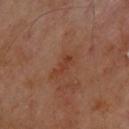Q: What did automated image analysis measure?
A: a footprint of about 3 mm², an eccentricity of roughly 0.9, and a symmetry-axis asymmetry near 0.45; an average lesion color of about L≈38 a*≈24 b*≈30 (CIELAB), about 6 CIELAB-L* units darker than the surrounding skin, and a normalized border contrast of about 6; border irregularity of about 5 on a 0–10 scale, internal color variation of about 0 on a 0–10 scale, and peripheral color asymmetry of about 0
Q: How was this image acquired?
A: total-body-photography crop, ~15 mm field of view
Q: Illumination type?
A: cross-polarized
Q: Patient demographics?
A: male, approximately 65 years of age
Q: Lesion size?
A: ≈3 mm
Q: Where on the body is the lesion?
A: the upper back